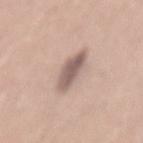Findings:
• follow-up — catalogued during a skin exam; not biopsied
• tile lighting — white-light illumination
• TBP lesion metrics — a footprint of about 8 mm², an outline eccentricity of about 0.9 (0 = round, 1 = elongated), and a symmetry-axis asymmetry near 0.15; a color-variation rating of about 3.5/10
• subject — female, aged 33–37
• imaging modality — 15 mm crop, total-body photography
• body site — the abdomen
• lesion size — ~5 mm (longest diameter)